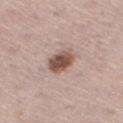Captured during whole-body skin photography for melanoma surveillance; the lesion was not biopsied. This is a white-light tile. The lesion is located on the right thigh. Measured at roughly 3.5 mm in maximum diameter. A roughly 15 mm field-of-view crop from a total-body skin photograph. The subject is a male about 65 years old.